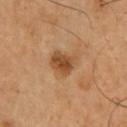Captured during whole-body skin photography for melanoma surveillance; the lesion was not biopsied. A 15 mm close-up extracted from a 3D total-body photography capture. The lesion is located on the chest. The patient is a male aged 48–52.